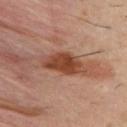The lesion was photographed on a routine skin check and not biopsied; there is no pathology result.
The lesion is located on the upper back.
An algorithmic analysis of the crop reported a footprint of about 11 mm², an outline eccentricity of about 0.85 (0 = round, 1 = elongated), and two-axis asymmetry of about 0.3. And it measured a mean CIELAB color near L≈43 a*≈24 b*≈31 and roughly 13 lightness units darker than nearby skin.
Approximately 5.5 mm at its widest.
A male patient, roughly 40 years of age.
Captured under cross-polarized illumination.
Cropped from a whole-body photographic skin survey; the tile spans about 15 mm.